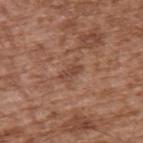{
  "biopsy_status": "not biopsied; imaged during a skin examination",
  "image": {
    "source": "total-body photography crop",
    "field_of_view_mm": 15
  },
  "lighting": "white-light",
  "patient": {
    "sex": "male",
    "age_approx": 75
  },
  "site": "upper back"
}